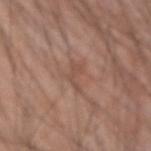Clinical impression: Part of a total-body skin-imaging series; this lesion was reviewed on a skin check and was not flagged for biopsy. Acquisition and patient details: An algorithmic analysis of the crop reported a footprint of about 3 mm², a shape eccentricity near 0.9, and a shape-asymmetry score of about 0.75 (0 = symmetric). Imaged with white-light lighting. The patient is a male in their mid- to late 40s. Cropped from a whole-body photographic skin survey; the tile spans about 15 mm. The lesion is located on the right forearm. About 3.5 mm across.